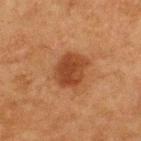Recorded during total-body skin imaging; not selected for excision or biopsy. Measured at roughly 4 mm in maximum diameter. A region of skin cropped from a whole-body photographic capture, roughly 15 mm wide. An algorithmic analysis of the crop reported a border-irregularity index near 1.5/10, internal color variation of about 3 on a 0–10 scale, and a peripheral color-asymmetry measure near 1. The analysis additionally found a classifier nevus-likeness of about 85/100 and lesion-presence confidence of about 100/100. The lesion is located on the upper back. A male patient in their mid- to late 70s.